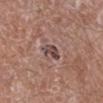A 15 mm close-up extracted from a 3D total-body photography capture. About 3 mm across. On the left lower leg. A male subject aged around 60. The tile uses white-light illumination.